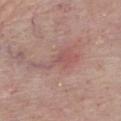Imaged during a routine full-body skin examination; the lesion was not biopsied and no histopathology is available. Longest diameter approximately 6.5 mm. Located on the chest. Captured under white-light illumination. A lesion tile, about 15 mm wide, cut from a 3D total-body photograph. The patient is a male aged 78 to 82.